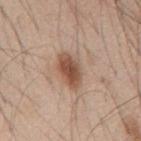The lesion was photographed on a routine skin check and not biopsied; there is no pathology result. The lesion is on the mid back. A 15 mm crop from a total-body photograph taken for skin-cancer surveillance. The lesion's longest dimension is about 4.5 mm. A male patient in their mid-40s.